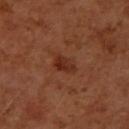Clinical impression: Recorded during total-body skin imaging; not selected for excision or biopsy. Clinical summary: Automated image analysis of the tile measured a footprint of about 4 mm², a shape eccentricity near 0.8, and a symmetry-axis asymmetry near 0.3. And it measured a mean CIELAB color near L≈31 a*≈26 b*≈30, a lesion–skin lightness drop of about 8, and a normalized lesion–skin contrast near 7.5. It also reported border irregularity of about 3 on a 0–10 scale, a within-lesion color-variation index near 2.5/10, and peripheral color asymmetry of about 1. The lesion's longest dimension is about 3 mm. Captured under cross-polarized illumination. The patient is a female about 50 years old. The lesion is located on the left forearm. A 15 mm close-up extracted from a 3D total-body photography capture.